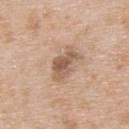Assessment: The lesion was photographed on a routine skin check and not biopsied; there is no pathology result. Background: A region of skin cropped from a whole-body photographic capture, roughly 15 mm wide. From the upper back. A male patient, aged approximately 65.